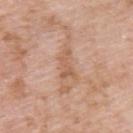Case summary:
• biopsy status · total-body-photography surveillance lesion; no biopsy
• image · total-body-photography crop, ~15 mm field of view
• location · the upper back
• patient · male, about 60 years old